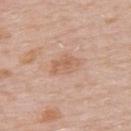| field | value |
|---|---|
| notes | catalogued during a skin exam; not biopsied |
| image | ~15 mm tile from a whole-body skin photo |
| lighting | white-light illumination |
| subject | male, approximately 75 years of age |
| diameter | ≈4 mm |
| image-analysis metrics | an average lesion color of about L≈62 a*≈20 b*≈31 (CIELAB), roughly 7 lightness units darker than nearby skin, and a normalized lesion–skin contrast near 5.5; border irregularity of about 3 on a 0–10 scale, internal color variation of about 3 on a 0–10 scale, and radial color variation of about 1; a classifier nevus-likeness of about 0/100 and lesion-presence confidence of about 100/100 |
| location | the upper back |